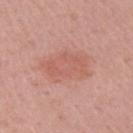Impression:
Imaged during a routine full-body skin examination; the lesion was not biopsied and no histopathology is available.
Clinical summary:
The tile uses white-light illumination. A close-up tile cropped from a whole-body skin photograph, about 15 mm across. A male patient, approximately 70 years of age. The lesion is on the right upper arm.